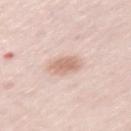biopsy status — no biopsy performed (imaged during a skin exam)
site — the left upper arm
lighting — white-light illumination
lesion size — ≈2.5 mm
subject — female, roughly 65 years of age
image-analysis metrics — a lesion-to-skin contrast of about 7 (normalized; higher = more distinct); a classifier nevus-likeness of about 60/100 and lesion-presence confidence of about 100/100
image — ~15 mm crop, total-body skin-cancer survey